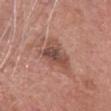biopsy status: catalogued during a skin exam; not biopsied | image-analysis metrics: an average lesion color of about L≈49 a*≈21 b*≈26 (CIELAB) and a normalized lesion–skin contrast near 7.5 | lesion diameter: ~4.5 mm (longest diameter) | anatomic site: the head or neck | patient: male, roughly 60 years of age | image: 15 mm crop, total-body photography.